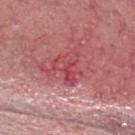Clinical impression: Recorded during total-body skin imaging; not selected for excision or biopsy. Background: A male patient aged approximately 70. A 15 mm crop from a total-body photograph taken for skin-cancer surveillance. The lesion is located on the head or neck. The tile uses white-light illumination. The recorded lesion diameter is about 4 mm.